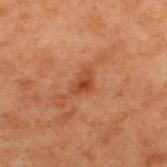A lesion tile, about 15 mm wide, cut from a 3D total-body photograph. Located on the upper back. A male patient, in their 70s. The total-body-photography lesion software estimated a footprint of about 4 mm², an outline eccentricity of about 0.75 (0 = round, 1 = elongated), and two-axis asymmetry of about 0.4. The analysis additionally found a nevus-likeness score of about 20/100 and a detector confidence of about 100 out of 100 that the crop contains a lesion. This is a cross-polarized tile. About 3 mm across.